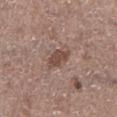Acquisition and patient details: From the left lower leg. A close-up tile cropped from a whole-body skin photograph, about 15 mm across. A male subject, in their mid- to late 60s. Imaged with white-light lighting.